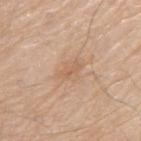The lesion was tiled from a total-body skin photograph and was not biopsied. The patient is a male aged approximately 80. An algorithmic analysis of the crop reported an automated nevus-likeness rating near 0 out of 100. The lesion is on the arm. A roughly 15 mm field-of-view crop from a total-body skin photograph. Longest diameter approximately 2.5 mm.